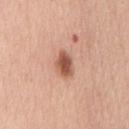Q: Was a biopsy performed?
A: catalogued during a skin exam; not biopsied
Q: What is the lesion's diameter?
A: about 3.5 mm
Q: What are the patient's age and sex?
A: male, aged 48 to 52
Q: How was this image acquired?
A: total-body-photography crop, ~15 mm field of view
Q: What lighting was used for the tile?
A: white-light illumination
Q: Lesion location?
A: the chest